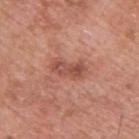| feature | finding |
|---|---|
| follow-up | imaged on a skin check; not biopsied |
| size | ~4 mm (longest diameter) |
| image source | 15 mm crop, total-body photography |
| lighting | white-light illumination |
| subject | male, aged 53 to 57 |
| location | the upper back |